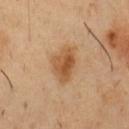Assessment:
Recorded during total-body skin imaging; not selected for excision or biopsy.
Background:
The lesion's longest dimension is about 4 mm. The lesion is located on the chest. A male subject aged around 50. Automated image analysis of the tile measured a nevus-likeness score of about 95/100 and lesion-presence confidence of about 100/100. Captured under cross-polarized illumination. A roughly 15 mm field-of-view crop from a total-body skin photograph.